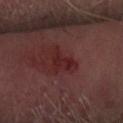Captured during whole-body skin photography for melanoma surveillance; the lesion was not biopsied.
A male subject aged around 65.
A 15 mm close-up tile from a total-body photography series done for melanoma screening.
From the right forearm.
The total-body-photography lesion software estimated an average lesion color of about L≈24 a*≈25 b*≈19 (CIELAB) and roughly 6 lightness units darker than nearby skin. It also reported a border-irregularity rating of about 6/10 and a peripheral color-asymmetry measure near 0.5.